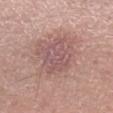Part of a total-body skin-imaging series; this lesion was reviewed on a skin check and was not flagged for biopsy. A region of skin cropped from a whole-body photographic capture, roughly 15 mm wide. The subject is a male roughly 60 years of age. The lesion is located on the right lower leg. Captured under white-light illumination. The recorded lesion diameter is about 4.5 mm.